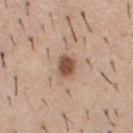Assessment: This lesion was catalogued during total-body skin photography and was not selected for biopsy. Image and clinical context: The tile uses white-light illumination. Longest diameter approximately 2.5 mm. From the chest. Automated image analysis of the tile measured a lesion area of about 5 mm², a shape eccentricity near 0.55, and a shape-asymmetry score of about 0.15 (0 = symmetric). The software also gave a lesion color around L≈51 a*≈20 b*≈28 in CIELAB and a lesion-to-skin contrast of about 10 (normalized; higher = more distinct). The software also gave a nevus-likeness score of about 95/100 and a detector confidence of about 100 out of 100 that the crop contains a lesion. A 15 mm crop from a total-body photograph taken for skin-cancer surveillance. A male patient aged 38–42.